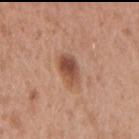  biopsy_status: not biopsied; imaged during a skin examination
  site: right upper arm
  image:
    source: total-body photography crop
    field_of_view_mm: 15
  patient:
    sex: male
    age_approx: 35
  lesion_size:
    long_diameter_mm_approx: 3.5